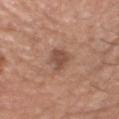Findings:
• biopsy status · imaged on a skin check; not biopsied
• size · ~3.5 mm (longest diameter)
• patient · male, aged approximately 75
• imaging modality · total-body-photography crop, ~15 mm field of view
• tile lighting · white-light
• body site · the head or neck